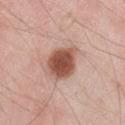No biopsy was performed on this lesion — it was imaged during a full skin examination and was not determined to be concerning. A roughly 15 mm field-of-view crop from a total-body skin photograph. The lesion is on the left thigh. Captured under white-light illumination. Measured at roughly 5 mm in maximum diameter. A male subject aged 43 to 47.